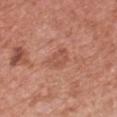biopsy_status: not biopsied; imaged during a skin examination
lighting: white-light
patient:
  sex: female
  age_approx: 50
site: chest
image:
  source: total-body photography crop
  field_of_view_mm: 15
lesion_size:
  long_diameter_mm_approx: 3.5